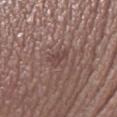The lesion was photographed on a routine skin check and not biopsied; there is no pathology result. The patient is a female aged approximately 30. A close-up tile cropped from a whole-body skin photograph, about 15 mm across. From the leg. The tile uses white-light illumination. Longest diameter approximately 2.5 mm.